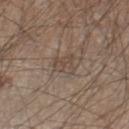<record>
<biopsy_status>not biopsied; imaged during a skin examination</biopsy_status>
<patient>
  <sex>male</sex>
  <age_approx>45</age_approx>
</patient>
<lesion_size>
  <long_diameter_mm_approx>3.0</long_diameter_mm_approx>
</lesion_size>
<lighting>white-light</lighting>
<site>right lower leg</site>
<automated_metrics>
  <eccentricity>0.55</eccentricity>
  <vs_skin_darker_L>6.0</vs_skin_darker_L>
  <vs_skin_contrast_norm>5.0</vs_skin_contrast_norm>
  <border_irregularity_0_10>4.0</border_irregularity_0_10>
  <peripheral_color_asymmetry>0.5</peripheral_color_asymmetry>
</automated_metrics>
<image>
  <source>total-body photography crop</source>
  <field_of_view_mm>15</field_of_view_mm>
</image>
</record>